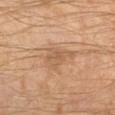{
  "biopsy_status": "not biopsied; imaged during a skin examination",
  "patient": {
    "sex": "male",
    "age_approx": 60
  },
  "image": {
    "source": "total-body photography crop",
    "field_of_view_mm": 15
  },
  "lesion_size": {
    "long_diameter_mm_approx": 4.5
  },
  "site": "left lower leg",
  "lighting": "cross-polarized"
}